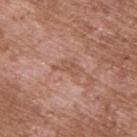A male subject, approximately 55 years of age. The tile uses white-light illumination. From the upper back. Measured at roughly 3.5 mm in maximum diameter. The lesion-visualizer software estimated a footprint of about 4.5 mm². The software also gave border irregularity of about 4.5 on a 0–10 scale, a within-lesion color-variation index near 1.5/10, and peripheral color asymmetry of about 0.5. The software also gave a nevus-likeness score of about 0/100 and a detector confidence of about 100 out of 100 that the crop contains a lesion. A close-up tile cropped from a whole-body skin photograph, about 15 mm across.